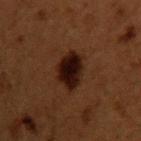Imaged during a routine full-body skin examination; the lesion was not biopsied and no histopathology is available.
A 15 mm close-up extracted from a 3D total-body photography capture.
The lesion's longest dimension is about 4 mm.
The lesion is on the upper back.
The patient is a male aged around 50.
Captured under cross-polarized illumination.
An algorithmic analysis of the crop reported a footprint of about 11 mm², a shape eccentricity near 0.55, and a shape-asymmetry score of about 0.15 (0 = symmetric). It also reported border irregularity of about 1.5 on a 0–10 scale and peripheral color asymmetry of about 1. The software also gave lesion-presence confidence of about 100/100.